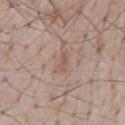Cropped from a whole-body photographic skin survey; the tile spans about 15 mm. The recorded lesion diameter is about 3 mm. A male patient, about 55 years old. Imaged with white-light lighting.